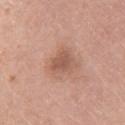The lesion was photographed on a routine skin check and not biopsied; there is no pathology result. A female subject, about 60 years old. A 15 mm close-up extracted from a 3D total-body photography capture. The lesion is located on the right upper arm.